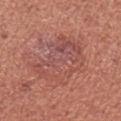Captured during whole-body skin photography for melanoma surveillance; the lesion was not biopsied.
Located on the right upper arm.
This image is a 15 mm lesion crop taken from a total-body photograph.
The patient is a female aged around 35.
The recorded lesion diameter is about 7.5 mm.